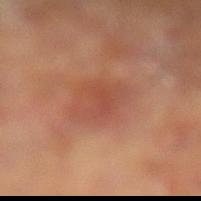Part of a total-body skin-imaging series; this lesion was reviewed on a skin check and was not flagged for biopsy.
The tile uses cross-polarized illumination.
A male subject, approximately 70 years of age.
Located on the left lower leg.
Measured at roughly 5 mm in maximum diameter.
An algorithmic analysis of the crop reported a lesion–skin lightness drop of about 6 and a lesion-to-skin contrast of about 4.5 (normalized; higher = more distinct).
A roughly 15 mm field-of-view crop from a total-body skin photograph.